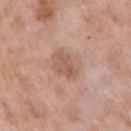| field | value |
|---|---|
| workup | no biopsy performed (imaged during a skin exam) |
| image | ~15 mm tile from a whole-body skin photo |
| image-analysis metrics | a footprint of about 7 mm² and a shape-asymmetry score of about 0.25 (0 = symmetric); an average lesion color of about L≈57 a*≈21 b*≈30 (CIELAB) and a normalized border contrast of about 6; a border-irregularity index near 3/10, a color-variation rating of about 3/10, and radial color variation of about 1; a classifier nevus-likeness of about 0/100 and a detector confidence of about 100 out of 100 that the crop contains a lesion |
| location | the right upper arm |
| size | about 3.5 mm |
| subject | female, aged around 70 |